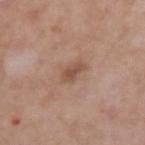{"biopsy_status": "not biopsied; imaged during a skin examination", "image": {"source": "total-body photography crop", "field_of_view_mm": 15}, "patient": {"sex": "male", "age_approx": 50}, "site": "left upper arm", "lesion_size": {"long_diameter_mm_approx": 2.5}, "lighting": "white-light", "automated_metrics": {"area_mm2_approx": 3.5, "eccentricity": 0.8, "shape_asymmetry": 0.3, "cielab_L": 51, "cielab_a": 20, "cielab_b": 29, "vs_skin_contrast_norm": 7.0, "lesion_detection_confidence_0_100": 100}}